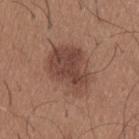A male patient, aged approximately 25.
The tile uses white-light illumination.
Automated tile analysis of the lesion measured a lesion area of about 23 mm² and an outline eccentricity of about 0.75 (0 = round, 1 = elongated). And it measured a mean CIELAB color near L≈45 a*≈20 b*≈26, about 10 CIELAB-L* units darker than the surrounding skin, and a normalized lesion–skin contrast near 7.5.
Cropped from a total-body skin-imaging series; the visible field is about 15 mm.
Measured at roughly 6.5 mm in maximum diameter.
On the upper back.